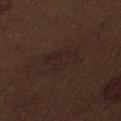Impression:
The lesion was photographed on a routine skin check and not biopsied; there is no pathology result.
Acquisition and patient details:
Automated tile analysis of the lesion measured an area of roughly 9 mm², an outline eccentricity of about 0.85 (0 = round, 1 = elongated), and a shape-asymmetry score of about 0.4 (0 = symmetric). On the abdomen. The tile uses white-light illumination. Cropped from a total-body skin-imaging series; the visible field is about 15 mm. Approximately 4.5 mm at its widest. A male patient, roughly 70 years of age.